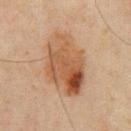Imaged during a routine full-body skin examination; the lesion was not biopsied and no histopathology is available. The patient is a male roughly 45 years of age. Imaged with cross-polarized lighting. A 15 mm close-up extracted from a 3D total-body photography capture. Approximately 7.5 mm at its widest. From the front of the torso.